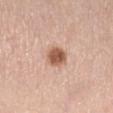notes — catalogued during a skin exam; not biopsied